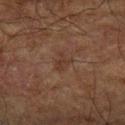No biopsy was performed on this lesion — it was imaged during a full skin examination and was not determined to be concerning.